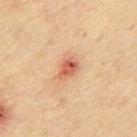Impression: Part of a total-body skin-imaging series; this lesion was reviewed on a skin check and was not flagged for biopsy. Context: The lesion's longest dimension is about 3 mm. Located on the chest. A lesion tile, about 15 mm wide, cut from a 3D total-body photograph. A male subject in their mid-60s. The total-body-photography lesion software estimated border irregularity of about 2.5 on a 0–10 scale. The analysis additionally found a nevus-likeness score of about 0/100 and a detector confidence of about 100 out of 100 that the crop contains a lesion. This is a cross-polarized tile.